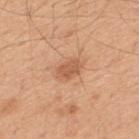The lesion was tiled from a total-body skin photograph and was not biopsied. A lesion tile, about 15 mm wide, cut from a 3D total-body photograph. A male patient, aged 48 to 52. Imaged with white-light lighting. From the upper back.